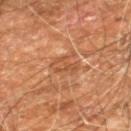workup: no biopsy performed (imaged during a skin exam); body site: the leg; subject: male, in their 60s; image: ~15 mm tile from a whole-body skin photo.